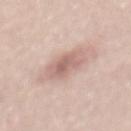| key | value |
|---|---|
| follow-up | total-body-photography surveillance lesion; no biopsy |
| imaging modality | ~15 mm tile from a whole-body skin photo |
| body site | the mid back |
| subject | male, in their 60s |